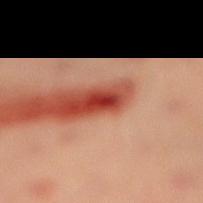{
  "biopsy_status": "not biopsied; imaged during a skin examination",
  "patient": {
    "sex": "female",
    "age_approx": 35
  },
  "site": "right lower leg",
  "image": {
    "source": "total-body photography crop",
    "field_of_view_mm": 15
  }
}